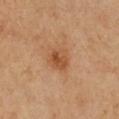A lesion tile, about 15 mm wide, cut from a 3D total-body photograph. Located on the front of the torso. The subject is a female aged 38–42.